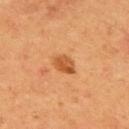Recorded during total-body skin imaging; not selected for excision or biopsy. A roughly 15 mm field-of-view crop from a total-body skin photograph. A male patient, in their mid-50s. From the upper back. The lesion's longest dimension is about 3 mm.